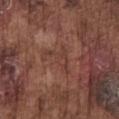notes: catalogued during a skin exam; not biopsied | lesion diameter: ≈4.5 mm | image-analysis metrics: a lesion color around L≈40 a*≈22 b*≈25 in CIELAB, a lesion–skin lightness drop of about 5, and a normalized lesion–skin contrast near 4.5; a border-irregularity index near 8/10, a within-lesion color-variation index near 1.5/10, and peripheral color asymmetry of about 0.5 | acquisition: ~15 mm tile from a whole-body skin photo | patient: male, in their mid- to late 70s | body site: the chest.